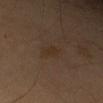Impression: No biopsy was performed on this lesion — it was imaged during a full skin examination and was not determined to be concerning.